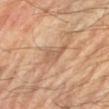Assessment: No biopsy was performed on this lesion — it was imaged during a full skin examination and was not determined to be concerning. Clinical summary: This image is a 15 mm lesion crop taken from a total-body photograph. Approximately 3.5 mm at its widest. Imaged with cross-polarized lighting. On the left forearm. A male patient, aged 68 to 72. An algorithmic analysis of the crop reported a lesion color around L≈54 a*≈18 b*≈32 in CIELAB, roughly 8 lightness units darker than nearby skin, and a lesion-to-skin contrast of about 5.5 (normalized; higher = more distinct).